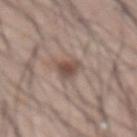Imaged during a routine full-body skin examination; the lesion was not biopsied and no histopathology is available. Measured at roughly 3 mm in maximum diameter. The total-body-photography lesion software estimated a lesion color around L≈48 a*≈17 b*≈23 in CIELAB, about 11 CIELAB-L* units darker than the surrounding skin, and a lesion-to-skin contrast of about 8.5 (normalized; higher = more distinct). The lesion is on the abdomen. A male subject roughly 45 years of age. A 15 mm crop from a total-body photograph taken for skin-cancer surveillance. This is a white-light tile.